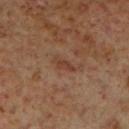Findings:
• workup · catalogued during a skin exam; not biopsied